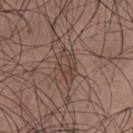No biopsy was performed on this lesion — it was imaged during a full skin examination and was not determined to be concerning. Located on the chest. The patient is a male aged approximately 35. Captured under white-light illumination. A 15 mm close-up extracted from a 3D total-body photography capture.